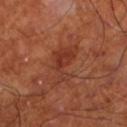Captured during whole-body skin photography for melanoma surveillance; the lesion was not biopsied. A close-up tile cropped from a whole-body skin photograph, about 15 mm across. Measured at roughly 5 mm in maximum diameter. Captured under cross-polarized illumination. Located on the left lower leg. A male patient about 70 years old.